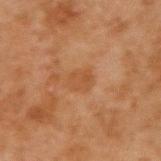biopsy status = catalogued during a skin exam; not biopsied | lesion size = ~2.5 mm (longest diameter) | anatomic site = the right upper arm | patient = male, aged 43 to 47 | illumination = cross-polarized | imaging modality = ~15 mm tile from a whole-body skin photo.